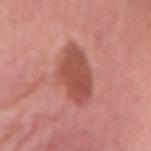notes = imaged on a skin check; not biopsied | imaging modality = ~15 mm crop, total-body skin-cancer survey | site = the abdomen | lesion size = ~5.5 mm (longest diameter) | illumination = white-light illumination | subject = female, roughly 75 years of age.